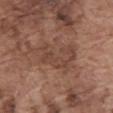Q: Is there a histopathology result?
A: imaged on a skin check; not biopsied
Q: How large is the lesion?
A: ~6 mm (longest diameter)
Q: What are the patient's age and sex?
A: male, aged around 75
Q: What is the anatomic site?
A: the front of the torso
Q: What kind of image is this?
A: ~15 mm crop, total-body skin-cancer survey
Q: Automated lesion metrics?
A: a footprint of about 14 mm², an outline eccentricity of about 0.85 (0 = round, 1 = elongated), and a symmetry-axis asymmetry near 0.4; an average lesion color of about L≈44 a*≈20 b*≈26 (CIELAB) and a lesion–skin lightness drop of about 7; border irregularity of about 6.5 on a 0–10 scale and internal color variation of about 3 on a 0–10 scale